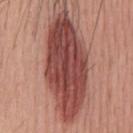Captured during whole-body skin photography for melanoma surveillance; the lesion was not biopsied. Imaged with white-light lighting. The recorded lesion diameter is about 13 mm. The total-body-photography lesion software estimated a footprint of about 47 mm² and a shape-asymmetry score of about 0.15 (0 = symmetric). The analysis additionally found a mean CIELAB color near L≈46 a*≈26 b*≈25, about 17 CIELAB-L* units darker than the surrounding skin, and a lesion-to-skin contrast of about 12 (normalized; higher = more distinct). The analysis additionally found a border-irregularity index near 2.5/10, a within-lesion color-variation index near 6/10, and peripheral color asymmetry of about 2. It also reported an automated nevus-likeness rating near 95 out of 100. Located on the upper back. This image is a 15 mm lesion crop taken from a total-body photograph. The patient is a male aged 43 to 47.